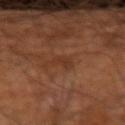notes: imaged on a skin check; not biopsied
anatomic site: the right forearm
size: ~2.5 mm (longest diameter)
subject: male, roughly 60 years of age
TBP lesion metrics: a border-irregularity rating of about 3/10, a within-lesion color-variation index near 0.5/10, and radial color variation of about 0; an automated nevus-likeness rating near 0 out of 100
imaging modality: ~15 mm crop, total-body skin-cancer survey